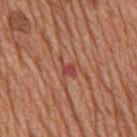Recorded during total-body skin imaging; not selected for excision or biopsy.
Located on the mid back.
Cropped from a whole-body photographic skin survey; the tile spans about 15 mm.
A male patient in their mid- to late 60s.